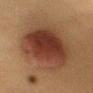Imaged during a routine full-body skin examination; the lesion was not biopsied and no histopathology is available.
Approximately 8 mm at its widest.
The tile uses cross-polarized illumination.
The subject is a female approximately 40 years of age.
An algorithmic analysis of the crop reported a lesion-detection confidence of about 100/100.
Cropped from a whole-body photographic skin survey; the tile spans about 15 mm.
On the right upper arm.